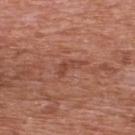Q: Was this lesion biopsied?
A: no biopsy performed (imaged during a skin exam)
Q: Lesion size?
A: ~3 mm (longest diameter)
Q: Lesion location?
A: the upper back
Q: What are the patient's age and sex?
A: male, aged 68–72
Q: What lighting was used for the tile?
A: white-light
Q: What kind of image is this?
A: ~15 mm crop, total-body skin-cancer survey